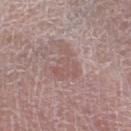biopsy_status: not biopsied; imaged during a skin examination
lighting: white-light
image:
  source: total-body photography crop
  field_of_view_mm: 15
patient:
  sex: male
  age_approx: 80
lesion_size:
  long_diameter_mm_approx: 4.5
site: right thigh